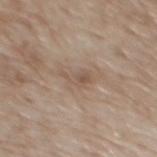No biopsy was performed on this lesion — it was imaged during a full skin examination and was not determined to be concerning.
Cropped from a whole-body photographic skin survey; the tile spans about 15 mm.
Located on the mid back.
A male subject aged around 70.
Imaged with white-light lighting.
The recorded lesion diameter is about 3 mm.